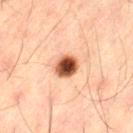No biopsy was performed on this lesion — it was imaged during a full skin examination and was not determined to be concerning. The lesion is on the left thigh. A male patient aged around 50. Approximately 3 mm at its widest. A roughly 15 mm field-of-view crop from a total-body skin photograph. An algorithmic analysis of the crop reported a lesion area of about 6.5 mm², a shape eccentricity near 0.3, and a symmetry-axis asymmetry near 0.1. The analysis additionally found a mean CIELAB color near L≈41 a*≈22 b*≈29 and a lesion–skin lightness drop of about 20. The analysis additionally found a border-irregularity rating of about 1/10, internal color variation of about 7.5 on a 0–10 scale, and a peripheral color-asymmetry measure near 2. This is a cross-polarized tile.